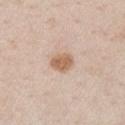No biopsy was performed on this lesion — it was imaged during a full skin examination and was not determined to be concerning. The subject is a male roughly 70 years of age. Automated tile analysis of the lesion measured an average lesion color of about L≈62 a*≈18 b*≈31 (CIELAB), a lesion–skin lightness drop of about 12, and a normalized lesion–skin contrast near 8. The analysis additionally found a color-variation rating of about 2.5/10 and a peripheral color-asymmetry measure near 1. And it measured a classifier nevus-likeness of about 90/100 and lesion-presence confidence of about 100/100. A 15 mm close-up extracted from a 3D total-body photography capture. The lesion's longest dimension is about 2.5 mm. Located on the right upper arm. The tile uses white-light illumination.